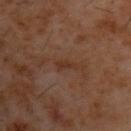Captured during whole-body skin photography for melanoma surveillance; the lesion was not biopsied. A male patient aged approximately 60. About 2.5 mm across. On the upper back. The total-body-photography lesion software estimated an area of roughly 2.5 mm², an eccentricity of roughly 0.9, and a shape-asymmetry score of about 0.45 (0 = symmetric). It also reported a border-irregularity index near 4.5/10 and a peripheral color-asymmetry measure near 0. Cropped from a total-body skin-imaging series; the visible field is about 15 mm.